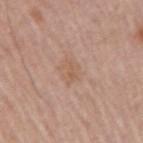The lesion was tiled from a total-body skin photograph and was not biopsied. The total-body-photography lesion software estimated roughly 6 lightness units darker than nearby skin and a normalized border contrast of about 5. The software also gave a border-irregularity index near 4/10, a within-lesion color-variation index near 1.5/10, and a peripheral color-asymmetry measure near 0.5. The software also gave a classifier nevus-likeness of about 0/100 and a lesion-detection confidence of about 100/100. The lesion is located on the right upper arm. The lesion's longest dimension is about 2.5 mm. This image is a 15 mm lesion crop taken from a total-body photograph. The subject is a male aged 53–57.